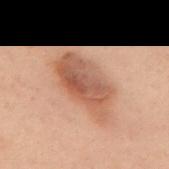<lesion>
  <biopsy_status>not biopsied; imaged during a skin examination</biopsy_status>
  <patient>
    <sex>female</sex>
    <age_approx>55</age_approx>
  </patient>
  <site>upper back</site>
  <image>
    <source>total-body photography crop</source>
    <field_of_view_mm>15</field_of_view_mm>
  </image>
</lesion>